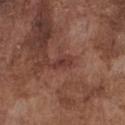Captured during whole-body skin photography for melanoma surveillance; the lesion was not biopsied. The lesion is on the chest. The lesion's longest dimension is about 2.5 mm. Automated image analysis of the tile measured a footprint of about 3 mm² and a shape eccentricity near 0.85. The analysis additionally found a lesion color around L≈37 a*≈23 b*≈24 in CIELAB, a lesion–skin lightness drop of about 8, and a normalized border contrast of about 7. It also reported a border-irregularity rating of about 3.5/10, internal color variation of about 1 on a 0–10 scale, and a peripheral color-asymmetry measure near 0.5. The analysis additionally found lesion-presence confidence of about 95/100. Captured under white-light illumination. A male subject in their mid- to late 70s. Cropped from a whole-body photographic skin survey; the tile spans about 15 mm.